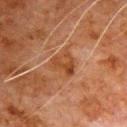Impression: This lesion was catalogued during total-body skin photography and was not selected for biopsy. Acquisition and patient details: A roughly 15 mm field-of-view crop from a total-body skin photograph. The lesion is located on the front of the torso. A male subject aged 78–82.